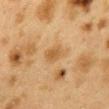{
  "biopsy_status": "not biopsied; imaged during a skin examination",
  "image": {
    "source": "total-body photography crop",
    "field_of_view_mm": 15
  },
  "site": "mid back",
  "patient": {
    "sex": "female",
    "age_approx": 40
  },
  "automated_metrics": {
    "cielab_L": 48,
    "cielab_a": 16,
    "cielab_b": 36,
    "vs_skin_darker_L": 7.0,
    "vs_skin_contrast_norm": 6.5
  },
  "lesion_size": {
    "long_diameter_mm_approx": 3.0
  }
}